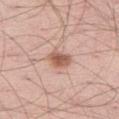Case summary:
• notes: catalogued during a skin exam; not biopsied
• image: ~15 mm tile from a whole-body skin photo
• subject: male, aged around 55
• body site: the right thigh
• lesion size: ~3 mm (longest diameter)
• illumination: white-light illumination